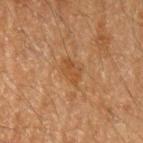Imaged during a routine full-body skin examination; the lesion was not biopsied and no histopathology is available. Located on the left upper arm. Cropped from a total-body skin-imaging series; the visible field is about 15 mm. Automated image analysis of the tile measured a lesion area of about 4 mm², an eccentricity of roughly 0.8, and two-axis asymmetry of about 0.35. The analysis additionally found a border-irregularity rating of about 3.5/10, internal color variation of about 1.5 on a 0–10 scale, and a peripheral color-asymmetry measure near 0.5. And it measured a nevus-likeness score of about 5/100 and lesion-presence confidence of about 100/100. The subject is a male approximately 65 years of age. Imaged with cross-polarized lighting.